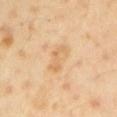patient: male, aged 43–47
tile lighting: cross-polarized illumination
image: 15 mm crop, total-body photography
automated lesion analysis: a footprint of about 7.5 mm² and a symmetry-axis asymmetry near 0.2; a mean CIELAB color near L≈69 a*≈17 b*≈39; a border-irregularity rating of about 2.5/10, a within-lesion color-variation index near 3/10, and radial color variation of about 1
lesion diameter: ~4 mm (longest diameter)
anatomic site: the left upper arm